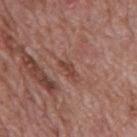| key | value |
|---|---|
| biopsy status | total-body-photography surveillance lesion; no biopsy |
| acquisition | 15 mm crop, total-body photography |
| lighting | white-light |
| subject | male, approximately 70 years of age |
| lesion size | about 2.5 mm |
| body site | the mid back |